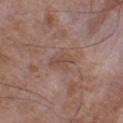Q: Is there a histopathology result?
A: catalogued during a skin exam; not biopsied
Q: Where on the body is the lesion?
A: the leg
Q: What lighting was used for the tile?
A: white-light illumination
Q: How was this image acquired?
A: ~15 mm tile from a whole-body skin photo
Q: What are the patient's age and sex?
A: male, aged around 50
Q: Lesion size?
A: ~3.5 mm (longest diameter)